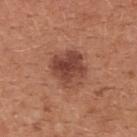  biopsy_status: not biopsied; imaged during a skin examination
  image:
    source: total-body photography crop
    field_of_view_mm: 15
  site: chest
  patient:
    sex: female
    age_approx: 35
  lighting: white-light